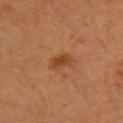On the chest. A close-up tile cropped from a whole-body skin photograph, about 15 mm across. A male patient, aged 48–52.